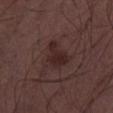  biopsy_status: not biopsied; imaged during a skin examination
  lesion_size:
    long_diameter_mm_approx: 3.5
  automated_metrics:
    area_mm2_approx: 6.0
    shape_asymmetry: 0.5
    cielab_L: 24
    cielab_a: 18
    cielab_b: 17
    vs_skin_darker_L: 7.0
    vs_skin_contrast_norm: 8.5
    border_irregularity_0_10: 5.0
    color_variation_0_10: 1.5
    peripheral_color_asymmetry: 0.5
  image:
    source: total-body photography crop
    field_of_view_mm: 15
  patient:
    sex: male
    age_approx: 50
  site: left lower leg
  lighting: white-light